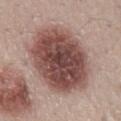The lesion was tiled from a total-body skin photograph and was not biopsied.
A close-up tile cropped from a whole-body skin photograph, about 15 mm across.
This is a white-light tile.
A female subject, aged 48 to 52.
Longest diameter approximately 9 mm.
An algorithmic analysis of the crop reported an eccentricity of roughly 0.6 and two-axis asymmetry of about 0.1. And it measured a mean CIELAB color near L≈47 a*≈20 b*≈22 and a normalized border contrast of about 12. It also reported a color-variation rating of about 6.5/10 and radial color variation of about 2.
The lesion is on the mid back.